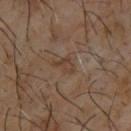Clinical impression: Recorded during total-body skin imaging; not selected for excision or biopsy. Image and clinical context: Imaged with cross-polarized lighting. An algorithmic analysis of the crop reported a lesion color around L≈36 a*≈15 b*≈25 in CIELAB and a normalized border contrast of about 5.5. The software also gave a border-irregularity rating of about 5/10 and radial color variation of about 0. A male subject aged around 65. Approximately 3 mm at its widest. From the upper back. A 15 mm close-up extracted from a 3D total-body photography capture.